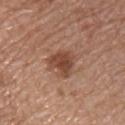Q: Is there a histopathology result?
A: no biopsy performed (imaged during a skin exam)
Q: How was the tile lit?
A: white-light
Q: How was this image acquired?
A: ~15 mm tile from a whole-body skin photo
Q: What is the anatomic site?
A: the chest
Q: Patient demographics?
A: male, in their mid- to late 50s
Q: Lesion size?
A: ~3.5 mm (longest diameter)
Q: What did automated image analysis measure?
A: an average lesion color of about L≈45 a*≈22 b*≈29 (CIELAB) and a lesion–skin lightness drop of about 11; a within-lesion color-variation index near 3/10 and peripheral color asymmetry of about 1